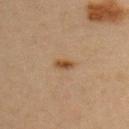The lesion was tiled from a total-body skin photograph and was not biopsied.
A female subject aged approximately 45.
This is a cross-polarized tile.
A lesion tile, about 15 mm wide, cut from a 3D total-body photograph.
The recorded lesion diameter is about 2.5 mm.
On the back.